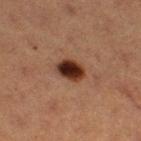Findings:
* diameter: ≈3.5 mm
* automated metrics: a lesion area of about 7 mm² and an eccentricity of roughly 0.65; a border-irregularity index near 2/10, a within-lesion color-variation index near 6/10, and a peripheral color-asymmetry measure near 1.5; a nevus-likeness score of about 100/100 and lesion-presence confidence of about 100/100
* acquisition: ~15 mm tile from a whole-body skin photo
* subject: female, about 40 years old
* body site: the right thigh
* tile lighting: cross-polarized